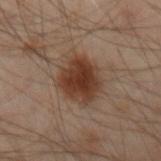Measured at roughly 4.5 mm in maximum diameter.
On the left arm.
A male patient, aged around 50.
A 15 mm close-up tile from a total-body photography series done for melanoma screening.
The lesion-visualizer software estimated a footprint of about 16 mm² and an outline eccentricity of about 0.6 (0 = round, 1 = elongated). The software also gave a lesion color around L≈30 a*≈16 b*≈23 in CIELAB, roughly 10 lightness units darker than nearby skin, and a lesion-to-skin contrast of about 10 (normalized; higher = more distinct). The software also gave internal color variation of about 4 on a 0–10 scale and radial color variation of about 1. The software also gave an automated nevus-likeness rating near 95 out of 100.